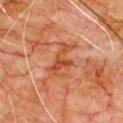Case summary:
• notes: no biopsy performed (imaged during a skin exam)
• image: total-body-photography crop, ~15 mm field of view
• tile lighting: cross-polarized
• patient: male, aged 78 to 82
• anatomic site: the chest
• size: ≈5.5 mm
• automated lesion analysis: a lesion area of about 10 mm² and a shape eccentricity near 0.9; an average lesion color of about L≈40 a*≈24 b*≈31 (CIELAB), about 7 CIELAB-L* units darker than the surrounding skin, and a lesion-to-skin contrast of about 6.5 (normalized; higher = more distinct); a border-irregularity rating of about 6/10, a color-variation rating of about 5/10, and a peripheral color-asymmetry measure near 1.5